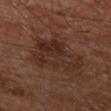notes: no biopsy performed (imaged during a skin exam); body site: the left thigh; lesion diameter: ~7 mm (longest diameter); image: ~15 mm crop, total-body skin-cancer survey; illumination: cross-polarized illumination; subject: male, roughly 65 years of age.